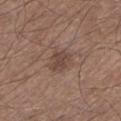Q: Was this lesion biopsied?
A: imaged on a skin check; not biopsied
Q: Automated lesion metrics?
A: a footprint of about 6 mm² and a shape eccentricity near 0.7; a border-irregularity rating of about 2.5/10, internal color variation of about 2 on a 0–10 scale, and a peripheral color-asymmetry measure near 0.5; an automated nevus-likeness rating near 5 out of 100 and a detector confidence of about 100 out of 100 that the crop contains a lesion
Q: What is the anatomic site?
A: the leg
Q: What are the patient's age and sex?
A: male, aged 73–77
Q: What is the lesion's diameter?
A: ~3.5 mm (longest diameter)
Q: How was the tile lit?
A: white-light illumination
Q: What kind of image is this?
A: total-body-photography crop, ~15 mm field of view